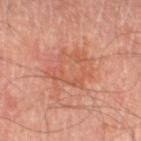Findings:
• workup · total-body-photography surveillance lesion; no biopsy
• TBP lesion metrics · an outline eccentricity of about 0.7 (0 = round, 1 = elongated) and two-axis asymmetry of about 0.25
• lesion diameter · ~6.5 mm (longest diameter)
• imaging modality · 15 mm crop, total-body photography
• subject · male, aged around 70
• site · the right thigh
• lighting · cross-polarized